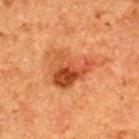notes: total-body-photography surveillance lesion; no biopsy | subject: male, aged approximately 50 | acquisition: 15 mm crop, total-body photography | body site: the back.